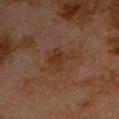No biopsy was performed on this lesion — it was imaged during a full skin examination and was not determined to be concerning.
This image is a 15 mm lesion crop taken from a total-body photograph.
Imaged with cross-polarized lighting.
The lesion is located on the chest.
A male patient, aged around 80.